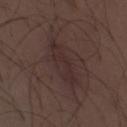This lesion was catalogued during total-body skin photography and was not selected for biopsy. The lesion is located on the chest. The total-body-photography lesion software estimated an area of roughly 9.5 mm², a shape eccentricity near 0.95, and a symmetry-axis asymmetry near 0.25. And it measured an average lesion color of about L≈28 a*≈15 b*≈16 (CIELAB), roughly 5 lightness units darker than nearby skin, and a normalized lesion–skin contrast near 6. And it measured a border-irregularity index near 4/10 and internal color variation of about 2 on a 0–10 scale. It also reported a nevus-likeness score of about 0/100. Approximately 6 mm at its widest. A 15 mm crop from a total-body photograph taken for skin-cancer surveillance. A male patient about 30 years old. The tile uses white-light illumination.